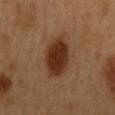A male patient, approximately 50 years of age.
A 15 mm crop from a total-body photograph taken for skin-cancer surveillance.
On the mid back.
Approximately 6.5 mm at its widest.
Automated image analysis of the tile measured a lesion color around L≈32 a*≈21 b*≈30 in CIELAB and about 13 CIELAB-L* units darker than the surrounding skin.